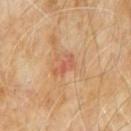| feature | finding |
|---|---|
| biopsy status | catalogued during a skin exam; not biopsied |
| illumination | cross-polarized illumination |
| patient | male, about 60 years old |
| anatomic site | the abdomen |
| acquisition | ~15 mm crop, total-body skin-cancer survey |
| lesion diameter | about 2.5 mm |